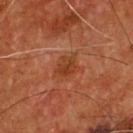Clinical impression:
No biopsy was performed on this lesion — it was imaged during a full skin examination and was not determined to be concerning.
Background:
A roughly 15 mm field-of-view crop from a total-body skin photograph. Automated image analysis of the tile measured a footprint of about 4 mm², a shape eccentricity near 0.6, and two-axis asymmetry of about 0.3. It also reported an automated nevus-likeness rating near 5 out of 100. About 2.5 mm across. A male subject about 55 years old. The lesion is located on the chest.